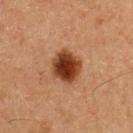biopsy status: total-body-photography surveillance lesion; no biopsy
illumination: cross-polarized
TBP lesion metrics: a nevus-likeness score of about 100/100 and lesion-presence confidence of about 100/100
diameter: ≈4 mm
patient: male, aged around 60
image source: ~15 mm crop, total-body skin-cancer survey